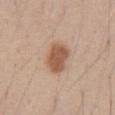The lesion is on the abdomen. The tile uses white-light illumination. The patient is a male roughly 40 years of age. The total-body-photography lesion software estimated a border-irregularity index near 2/10, a within-lesion color-variation index near 2.5/10, and a peripheral color-asymmetry measure near 1. And it measured a lesion-detection confidence of about 100/100. A 15 mm close-up tile from a total-body photography series done for melanoma screening.